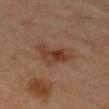Case summary:
• notes · catalogued during a skin exam; not biopsied
• site · the right forearm
• subject · female, about 60 years old
• diameter · ≈5 mm
• automated metrics · an average lesion color of about L≈39 a*≈20 b*≈29 (CIELAB) and a normalized lesion–skin contrast near 7.5; a classifier nevus-likeness of about 80/100
• image · 15 mm crop, total-body photography